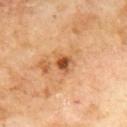The lesion was photographed on a routine skin check and not biopsied; there is no pathology result. The lesion is located on the mid back. This is a cross-polarized tile. A close-up tile cropped from a whole-body skin photograph, about 15 mm across. A male patient, approximately 70 years of age. Automated image analysis of the tile measured a within-lesion color-variation index near 4/10 and peripheral color asymmetry of about 1.5. And it measured a classifier nevus-likeness of about 60/100 and a detector confidence of about 100 out of 100 that the crop contains a lesion.